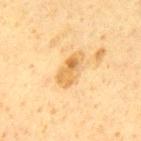notes — imaged on a skin check; not biopsied
image-analysis metrics — a lesion area of about 8.5 mm², a shape eccentricity near 0.85, and two-axis asymmetry of about 0.2; a border-irregularity index near 2/10, internal color variation of about 6 on a 0–10 scale, and radial color variation of about 2
image — 15 mm crop, total-body photography
location — the mid back
tile lighting — cross-polarized illumination
patient — male, roughly 60 years of age
size — about 4.5 mm